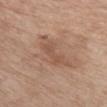Part of a total-body skin-imaging series; this lesion was reviewed on a skin check and was not flagged for biopsy.
The lesion is located on the chest.
This is a white-light tile.
The total-body-photography lesion software estimated an area of roughly 9.5 mm², an outline eccentricity of about 0.9 (0 = round, 1 = elongated), and a symmetry-axis asymmetry near 0.45. It also reported about 8 CIELAB-L* units darker than the surrounding skin and a lesion-to-skin contrast of about 5.5 (normalized; higher = more distinct). And it measured a border-irregularity index near 6/10, a within-lesion color-variation index near 3/10, and peripheral color asymmetry of about 1. And it measured a detector confidence of about 100 out of 100 that the crop contains a lesion.
A female patient, approximately 80 years of age.
Cropped from a whole-body photographic skin survey; the tile spans about 15 mm.
Measured at roughly 6 mm in maximum diameter.